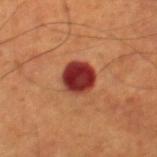– notes · total-body-photography surveillance lesion; no biopsy
– acquisition · 15 mm crop, total-body photography
– subject · male, aged around 70
– image-analysis metrics · a border-irregularity index near 1/10, internal color variation of about 6 on a 0–10 scale, and peripheral color asymmetry of about 2; lesion-presence confidence of about 100/100
– lighting · cross-polarized illumination
– anatomic site · the right thigh
– lesion diameter · ~3.5 mm (longest diameter)